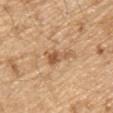| key | value |
|---|---|
| workup | no biopsy performed (imaged during a skin exam) |
| lighting | white-light illumination |
| patient | male, aged 68–72 |
| anatomic site | the chest |
| image source | ~15 mm crop, total-body skin-cancer survey |